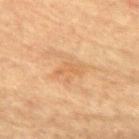Imaged during a routine full-body skin examination; the lesion was not biopsied and no histopathology is available.
The recorded lesion diameter is about 3 mm.
Captured under cross-polarized illumination.
The subject is a female aged 78 to 82.
The lesion-visualizer software estimated a footprint of about 2.5 mm² and a symmetry-axis asymmetry near 0.5. The software also gave a border-irregularity index near 5.5/10, internal color variation of about 0 on a 0–10 scale, and a peripheral color-asymmetry measure near 0.
A 15 mm close-up tile from a total-body photography series done for melanoma screening.
Located on the upper back.